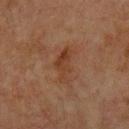Captured during whole-body skin photography for melanoma surveillance; the lesion was not biopsied. A male patient, in their mid- to late 60s. On the upper back. A 15 mm close-up extracted from a 3D total-body photography capture.